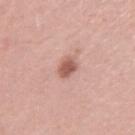Part of a total-body skin-imaging series; this lesion was reviewed on a skin check and was not flagged for biopsy.
The lesion is on the left upper arm.
About 2.5 mm across.
The total-body-photography lesion software estimated an automated nevus-likeness rating near 90 out of 100 and lesion-presence confidence of about 100/100.
A lesion tile, about 15 mm wide, cut from a 3D total-body photograph.
A female subject aged around 35.